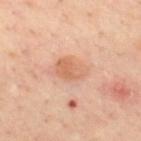The subject is a male roughly 45 years of age. Located on the upper back. This image is a 15 mm lesion crop taken from a total-body photograph. Approximately 4 mm at its widest. The tile uses cross-polarized illumination.